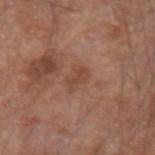biopsy status: imaged on a skin check; not biopsied
image: total-body-photography crop, ~15 mm field of view
tile lighting: white-light
diameter: ~3 mm (longest diameter)
subject: male, aged approximately 65
site: the right upper arm
automated lesion analysis: an average lesion color of about L≈46 a*≈22 b*≈28 (CIELAB) and a lesion-to-skin contrast of about 5 (normalized; higher = more distinct); a color-variation rating of about 2/10 and a peripheral color-asymmetry measure near 1; an automated nevus-likeness rating near 0 out of 100 and lesion-presence confidence of about 100/100